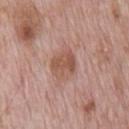Q: What is the anatomic site?
A: the front of the torso
Q: What are the patient's age and sex?
A: male, aged approximately 70
Q: What kind of image is this?
A: ~15 mm crop, total-body skin-cancer survey
Q: What is the lesion's diameter?
A: about 4 mm
Q: Illumination type?
A: white-light illumination